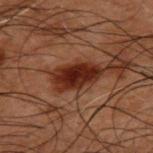| field | value |
|---|---|
| notes | catalogued during a skin exam; not biopsied |
| subject | male, aged 48–52 |
| lighting | cross-polarized illumination |
| imaging modality | ~15 mm crop, total-body skin-cancer survey |
| TBP lesion metrics | a footprint of about 11 mm², a shape eccentricity near 0.85, and a symmetry-axis asymmetry near 0.2; border irregularity of about 2 on a 0–10 scale, a within-lesion color-variation index near 4/10, and a peripheral color-asymmetry measure near 1 |
| body site | the upper back |